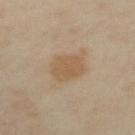follow-up = no biopsy performed (imaged during a skin exam).